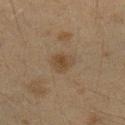Q: Was this lesion biopsied?
A: imaged on a skin check; not biopsied
Q: Lesion size?
A: ≈2.5 mm
Q: How was this image acquired?
A: total-body-photography crop, ~15 mm field of view
Q: Illumination type?
A: cross-polarized
Q: What is the anatomic site?
A: the left upper arm
Q: Patient demographics?
A: female, in their 40s